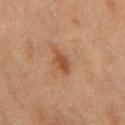About 2.5 mm across.
A male subject, aged around 75.
A close-up tile cropped from a whole-body skin photograph, about 15 mm across.
Imaged with cross-polarized lighting.
The lesion is on the mid back.